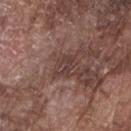imaging modality=~15 mm crop, total-body skin-cancer survey
subject=male, aged around 75
body site=the right forearm